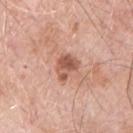workup: total-body-photography surveillance lesion; no biopsy
location: the chest
image source: total-body-photography crop, ~15 mm field of view
patient: male, roughly 70 years of age